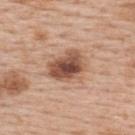<lesion>
  <biopsy_status>not biopsied; imaged during a skin examination</biopsy_status>
</lesion>